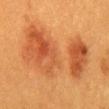{
  "biopsy_status": "not biopsied; imaged during a skin examination",
  "automated_metrics": {
    "eccentricity": 0.85,
    "shape_asymmetry": 0.35,
    "cielab_L": 46,
    "cielab_a": 24,
    "cielab_b": 36,
    "vs_skin_darker_L": 8.0,
    "vs_skin_contrast_norm": 6.5,
    "border_irregularity_0_10": 5.5,
    "lesion_detection_confidence_0_100": 100
  },
  "image": {
    "source": "total-body photography crop",
    "field_of_view_mm": 15
  },
  "lesion_size": {
    "long_diameter_mm_approx": 11.0
  },
  "lighting": "cross-polarized",
  "patient": {
    "sex": "female",
    "age_approx": 40
  },
  "site": "mid back"
}